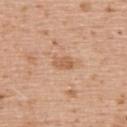Imaged during a routine full-body skin examination; the lesion was not biopsied and no histopathology is available. A male patient, approximately 70 years of age. A 15 mm close-up tile from a total-body photography series done for melanoma screening. From the back.